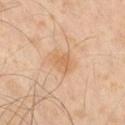  site: right thigh
  patient:
    sex: male
    age_approx: 60
  image:
    source: total-body photography crop
    field_of_view_mm: 15